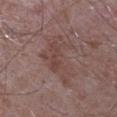{"biopsy_status": "not biopsied; imaged during a skin examination", "automated_metrics": {"eccentricity": 0.85, "shape_asymmetry": 0.55, "border_irregularity_0_10": 8.0, "color_variation_0_10": 3.5, "peripheral_color_asymmetry": 1.0, "nevus_likeness_0_100": 0, "lesion_detection_confidence_0_100": 100}, "patient": {"sex": "male", "age_approx": 65}, "lesion_size": {"long_diameter_mm_approx": 7.0}, "image": {"source": "total-body photography crop", "field_of_view_mm": 15}, "site": "left upper arm"}